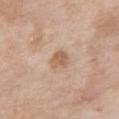Context: The tile uses white-light illumination. The lesion-visualizer software estimated an area of roughly 4.5 mm² and an eccentricity of roughly 0.6. It also reported a mean CIELAB color near L≈60 a*≈18 b*≈31 and roughly 10 lightness units darker than nearby skin. The lesion is on the chest. A female subject in their mid- to late 70s. A region of skin cropped from a whole-body photographic capture, roughly 15 mm wide.